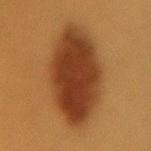The lesion's longest dimension is about 10 mm. A female subject about 35 years old. Imaged with cross-polarized lighting. On the lower back. A 15 mm crop from a total-body photograph taken for skin-cancer surveillance. Automated image analysis of the tile measured a lesion area of about 43 mm², an outline eccentricity of about 0.8 (0 = round, 1 = elongated), and a shape-asymmetry score of about 0.1 (0 = symmetric). And it measured about 13 CIELAB-L* units darker than the surrounding skin and a normalized lesion–skin contrast near 12. And it measured a nevus-likeness score of about 100/100 and a detector confidence of about 100 out of 100 that the crop contains a lesion. On biopsy, histopathology showed a dysplastic (Clark) nevus.